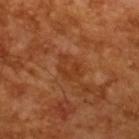A 15 mm crop from a total-body photograph taken for skin-cancer surveillance. Measured at roughly 3.5 mm in maximum diameter. The tile uses cross-polarized illumination. A male patient, aged approximately 65. An algorithmic analysis of the crop reported border irregularity of about 6.5 on a 0–10 scale, a within-lesion color-variation index near 0.5/10, and peripheral color asymmetry of about 0. And it measured a lesion-detection confidence of about 100/100.